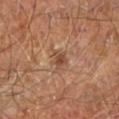<case>
<biopsy_status>not biopsied; imaged during a skin examination</biopsy_status>
<lesion_size>
  <long_diameter_mm_approx>2.5</long_diameter_mm_approx>
</lesion_size>
<patient>
  <sex>male</sex>
  <age_approx>60</age_approx>
</patient>
<site>right leg</site>
<image>
  <source>total-body photography crop</source>
  <field_of_view_mm>15</field_of_view_mm>
</image>
</case>